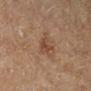Captured during whole-body skin photography for melanoma surveillance; the lesion was not biopsied.
The lesion-visualizer software estimated a lesion area of about 4 mm², an outline eccentricity of about 0.6 (0 = round, 1 = elongated), and a shape-asymmetry score of about 0.4 (0 = symmetric). The analysis additionally found an average lesion color of about L≈42 a*≈18 b*≈28 (CIELAB) and a lesion–skin lightness drop of about 7.
Measured at roughly 2.5 mm in maximum diameter.
Imaged with cross-polarized lighting.
A 15 mm crop from a total-body photograph taken for skin-cancer surveillance.
A female patient aged 63 to 67.
From the right lower leg.